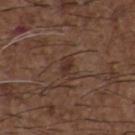<case>
<biopsy_status>not biopsied; imaged during a skin examination</biopsy_status>
<patient>
  <sex>male</sex>
  <age_approx>50</age_approx>
</patient>
<automated_metrics>
  <cielab_L>32</cielab_L>
  <cielab_a>17</cielab_a>
  <cielab_b>23</cielab_b>
  <vs_skin_darker_L>6.0</vs_skin_darker_L>
  <vs_skin_contrast_norm>6.5</vs_skin_contrast_norm>
  <border_irregularity_0_10>3.5</border_irregularity_0_10>
  <color_variation_0_10>2.0</color_variation_0_10>
  <peripheral_color_asymmetry>0.5</peripheral_color_asymmetry>
  <nevus_likeness_0_100>0</nevus_likeness_0_100>
  <lesion_detection_confidence_0_100>95</lesion_detection_confidence_0_100>
</automated_metrics>
<site>upper back</site>
<lesion_size>
  <long_diameter_mm_approx>3.5</long_diameter_mm_approx>
</lesion_size>
<image>
  <source>total-body photography crop</source>
  <field_of_view_mm>15</field_of_view_mm>
</image>
</case>